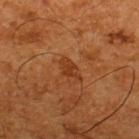Q: Was a biopsy performed?
A: total-body-photography surveillance lesion; no biopsy
Q: What are the patient's age and sex?
A: male, roughly 65 years of age
Q: How was this image acquired?
A: total-body-photography crop, ~15 mm field of view
Q: Where on the body is the lesion?
A: the upper back
Q: Automated lesion metrics?
A: a shape eccentricity near 0.8 and a shape-asymmetry score of about 0.4 (0 = symmetric); an average lesion color of about L≈30 a*≈23 b*≈32 (CIELAB), a lesion–skin lightness drop of about 7, and a normalized lesion–skin contrast near 6.5; a classifier nevus-likeness of about 0/100 and a detector confidence of about 100 out of 100 that the crop contains a lesion